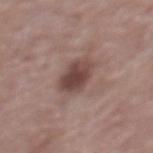Assessment:
No biopsy was performed on this lesion — it was imaged during a full skin examination and was not determined to be concerning.
Background:
A female patient, aged 63 to 67. Imaged with white-light lighting. An algorithmic analysis of the crop reported a footprint of about 8.5 mm², a shape eccentricity near 0.55, and two-axis asymmetry of about 0.15. It also reported a border-irregularity rating of about 1.5/10 and a within-lesion color-variation index near 4/10. Approximately 3.5 mm at its widest. From the back. A close-up tile cropped from a whole-body skin photograph, about 15 mm across.